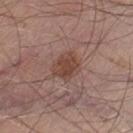notes = no biopsy performed (imaged during a skin exam) | automated lesion analysis = a mean CIELAB color near L≈44 a*≈20 b*≈25 and a normalized lesion–skin contrast near 7.5; a border-irregularity index near 2/10, a within-lesion color-variation index near 2.5/10, and a peripheral color-asymmetry measure near 1; a classifier nevus-likeness of about 85/100 | anatomic site = the left thigh | lighting = white-light illumination | imaging modality = 15 mm crop, total-body photography | patient = male, in their mid- to late 40s.